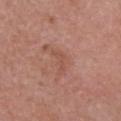Imaged during a routine full-body skin examination; the lesion was not biopsied and no histopathology is available. The total-body-photography lesion software estimated an average lesion color of about L≈52 a*≈24 b*≈28 (CIELAB), a lesion–skin lightness drop of about 6, and a normalized border contrast of about 4.5. It also reported border irregularity of about 5.5 on a 0–10 scale, a color-variation rating of about 0/10, and peripheral color asymmetry of about 0. The analysis additionally found a nevus-likeness score of about 0/100. Located on the head or neck. The subject is a male aged 68–72. Captured under white-light illumination. A lesion tile, about 15 mm wide, cut from a 3D total-body photograph. The lesion's longest dimension is about 3 mm.